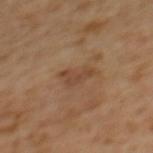Case summary:
* notes — no biopsy performed (imaged during a skin exam)
* image-analysis metrics — a shape eccentricity near 0.85 and a symmetry-axis asymmetry near 0.5; a mean CIELAB color near L≈45 a*≈19 b*≈32 and a normalized lesion–skin contrast near 6; a nevus-likeness score of about 0/100
* anatomic site — the back
* subject — female, in their mid- to late 50s
* diameter — ≈3.5 mm
* illumination — cross-polarized
* image — 15 mm crop, total-body photography